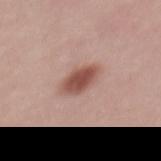Q: Was a biopsy performed?
A: imaged on a skin check; not biopsied
Q: What is the anatomic site?
A: the mid back
Q: Patient demographics?
A: female, aged 23 to 27
Q: How was this image acquired?
A: ~15 mm tile from a whole-body skin photo
Q: What did automated image analysis measure?
A: a footprint of about 7.5 mm², an eccentricity of roughly 0.85, and a symmetry-axis asymmetry near 0.2; an average lesion color of about L≈51 a*≈23 b*≈25 (CIELAB) and roughly 14 lightness units darker than nearby skin; a border-irregularity index near 2.5/10 and a peripheral color-asymmetry measure near 1; a classifier nevus-likeness of about 100/100 and a detector confidence of about 100 out of 100 that the crop contains a lesion
Q: Lesion size?
A: about 4 mm
Q: How was the tile lit?
A: white-light illumination